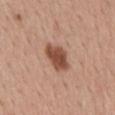Assessment:
The lesion was photographed on a routine skin check and not biopsied; there is no pathology result.
Background:
Automated tile analysis of the lesion measured a mean CIELAB color near L≈49 a*≈23 b*≈29 and roughly 15 lightness units darker than nearby skin. And it measured a border-irregularity index near 2.5/10 and internal color variation of about 2.5 on a 0–10 scale. A female patient roughly 55 years of age. From the mid back. A roughly 15 mm field-of-view crop from a total-body skin photograph. This is a white-light tile.